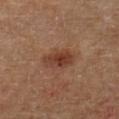Acquisition and patient details:
Cropped from a total-body skin-imaging series; the visible field is about 15 mm. Located on the left lower leg. The total-body-photography lesion software estimated a lesion color around L≈40 a*≈22 b*≈29 in CIELAB and about 9 CIELAB-L* units darker than the surrounding skin. It also reported a nevus-likeness score of about 85/100 and a lesion-detection confidence of about 100/100. A male subject aged approximately 65. About 4 mm across.